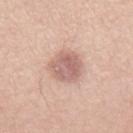The lesion was tiled from a total-body skin photograph and was not biopsied. The tile uses white-light illumination. A female patient, roughly 60 years of age. An algorithmic analysis of the crop reported a lesion–skin lightness drop of about 11. On the lower back. A 15 mm close-up extracted from a 3D total-body photography capture.